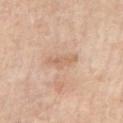Background: The subject is a female about 70 years old. The lesion is located on the arm. Automated image analysis of the tile measured a mean CIELAB color near L≈64 a*≈18 b*≈32, a lesion–skin lightness drop of about 8, and a normalized border contrast of about 5.5. The software also gave a border-irregularity index near 4.5/10, a within-lesion color-variation index near 2/10, and peripheral color asymmetry of about 0.5. The analysis additionally found a nevus-likeness score of about 0/100 and a lesion-detection confidence of about 100/100. A roughly 15 mm field-of-view crop from a total-body skin photograph. About 3.5 mm across. The tile uses white-light illumination.